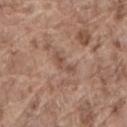Q: What lighting was used for the tile?
A: white-light illumination
Q: Automated lesion metrics?
A: a color-variation rating of about 0/10 and peripheral color asymmetry of about 0; a lesion-detection confidence of about 90/100
Q: Lesion location?
A: the lower back
Q: Who is the patient?
A: male, aged approximately 80
Q: What is the lesion's diameter?
A: about 3.5 mm
Q: What kind of image is this?
A: total-body-photography crop, ~15 mm field of view